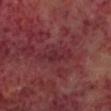| feature | finding |
|---|---|
| follow-up | imaged on a skin check; not biopsied |
| imaging modality | ~15 mm crop, total-body skin-cancer survey |
| anatomic site | the head or neck |
| subject | male, aged around 60 |
| lesion size | ~3.5 mm (longest diameter) |
| lighting | cross-polarized |
| image-analysis metrics | a lesion color around L≈28 a*≈28 b*≈16 in CIELAB, a lesion–skin lightness drop of about 5, and a normalized border contrast of about 5.5; border irregularity of about 3.5 on a 0–10 scale and a within-lesion color-variation index near 2/10 |